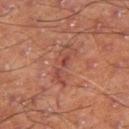Q: Was this lesion biopsied?
A: catalogued during a skin exam; not biopsied
Q: Who is the patient?
A: male, approximately 60 years of age
Q: Lesion size?
A: ~5 mm (longest diameter)
Q: How was the tile lit?
A: cross-polarized illumination
Q: How was this image acquired?
A: ~15 mm crop, total-body skin-cancer survey
Q: Lesion location?
A: the right thigh